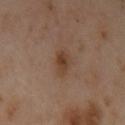Clinical impression:
Recorded during total-body skin imaging; not selected for excision or biopsy.
Clinical summary:
The lesion is on the arm. The recorded lesion diameter is about 3 mm. A region of skin cropped from a whole-body photographic capture, roughly 15 mm wide. A female patient, aged around 40.